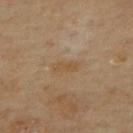| feature | finding |
|---|---|
| biopsy status | imaged on a skin check; not biopsied |
| patient | female, approximately 70 years of age |
| location | the upper back |
| automated lesion analysis | a lesion area of about 3.5 mm², a shape eccentricity near 0.9, and a symmetry-axis asymmetry near 0.25; an average lesion color of about L≈52 a*≈16 b*≈36 (CIELAB), about 5 CIELAB-L* units darker than the surrounding skin, and a lesion-to-skin contrast of about 6 (normalized; higher = more distinct); a classifier nevus-likeness of about 0/100 |
| imaging modality | ~15 mm tile from a whole-body skin photo |
| diameter | about 3 mm |